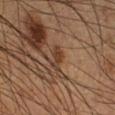follow-up=total-body-photography surveillance lesion; no biopsy | patient=male, aged around 60 | acquisition=total-body-photography crop, ~15 mm field of view | location=the right lower leg.